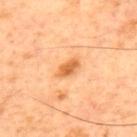biopsy status=imaged on a skin check; not biopsied
patient=male, aged 58 to 62
anatomic site=the back
image=~15 mm crop, total-body skin-cancer survey